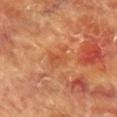* workup · total-body-photography surveillance lesion; no biopsy
* TBP lesion metrics · a footprint of about 5 mm² and two-axis asymmetry of about 0.4
* lighting · cross-polarized
* image source · ~15 mm crop, total-body skin-cancer survey
* subject · female, aged 78 to 82
* size · ≈3 mm
* anatomic site · the chest